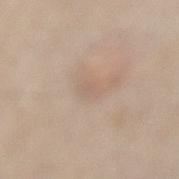Q: Was this lesion biopsied?
A: imaged on a skin check; not biopsied
Q: How was this image acquired?
A: ~15 mm crop, total-body skin-cancer survey
Q: What did automated image analysis measure?
A: a border-irregularity index near 2/10 and a color-variation rating of about 0/10; an automated nevus-likeness rating near 0 out of 100 and a lesion-detection confidence of about 85/100
Q: Lesion size?
A: ~2 mm (longest diameter)
Q: Lesion location?
A: the lower back
Q: Who is the patient?
A: female, in their mid- to late 60s